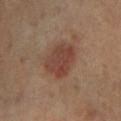notes: no biopsy performed (imaged during a skin exam) | automated lesion analysis: a footprint of about 13 mm², a shape eccentricity near 0.6, and a symmetry-axis asymmetry near 0.15; an average lesion color of about L≈39 a*≈19 b*≈25 (CIELAB) and roughly 8 lightness units darker than nearby skin; an automated nevus-likeness rating near 75 out of 100 and a detector confidence of about 100 out of 100 that the crop contains a lesion | location: the leg | image source: ~15 mm crop, total-body skin-cancer survey | lighting: cross-polarized | patient: male, aged around 65.